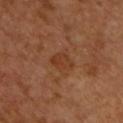* workup: imaged on a skin check; not biopsied
* anatomic site: the chest
* size: ~3 mm (longest diameter)
* image: ~15 mm crop, total-body skin-cancer survey
* illumination: cross-polarized
* patient: male, in their 50s